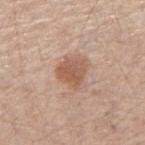Case summary:
• workup: no biopsy performed (imaged during a skin exam)
• TBP lesion metrics: a lesion area of about 8.5 mm², an outline eccentricity of about 0.35 (0 = round, 1 = elongated), and a symmetry-axis asymmetry near 0.3; an automated nevus-likeness rating near 95 out of 100 and a detector confidence of about 100 out of 100 that the crop contains a lesion
• lighting: white-light illumination
• lesion diameter: ~3.5 mm (longest diameter)
• patient: male, aged around 60
• body site: the right upper arm
• acquisition: ~15 mm crop, total-body skin-cancer survey